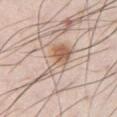Impression:
The lesion was photographed on a routine skin check and not biopsied; there is no pathology result.